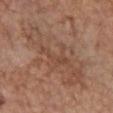  biopsy_status: not biopsied; imaged during a skin examination
  image:
    source: total-body photography crop
    field_of_view_mm: 15
  patient:
    sex: female
    age_approx: 65
  site: chest